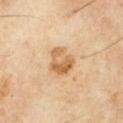follow-up: total-body-photography surveillance lesion; no biopsy
diameter: about 3.5 mm
site: the chest
patient: male, aged around 70
TBP lesion metrics: a lesion area of about 8 mm², an eccentricity of roughly 0.6, and a symmetry-axis asymmetry near 0.25; a within-lesion color-variation index near 7/10 and radial color variation of about 2.5
image source: total-body-photography crop, ~15 mm field of view